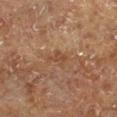This lesion was catalogued during total-body skin photography and was not selected for biopsy. A male subject in their mid-70s. An algorithmic analysis of the crop reported an automated nevus-likeness rating near 0 out of 100 and lesion-presence confidence of about 100/100. From the right lower leg. The tile uses cross-polarized illumination. A 15 mm close-up tile from a total-body photography series done for melanoma screening.